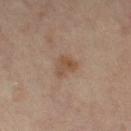{"biopsy_status": "not biopsied; imaged during a skin examination", "lighting": "cross-polarized", "image": {"source": "total-body photography crop", "field_of_view_mm": 15}, "lesion_size": {"long_diameter_mm_approx": 2.5}, "site": "right leg", "patient": {"sex": "female", "age_approx": 65}}